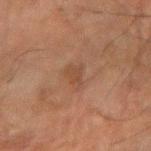notes = catalogued during a skin exam; not biopsied
tile lighting = cross-polarized illumination
lesion diameter = about 3 mm
acquisition = total-body-photography crop, ~15 mm field of view
patient = male, aged 63 to 67
location = the arm